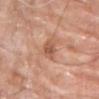diameter = ~2.5 mm (longest diameter)
image source = 15 mm crop, total-body photography
site = the chest
patient = male, approximately 65 years of age